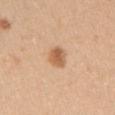<lesion>
<biopsy_status>not biopsied; imaged during a skin examination</biopsy_status>
<lesion_size>
  <long_diameter_mm_approx>3.0</long_diameter_mm_approx>
</lesion_size>
<site>left upper arm</site>
<lighting>white-light</lighting>
<image>
  <source>total-body photography crop</source>
  <field_of_view_mm>15</field_of_view_mm>
</image>
<patient>
  <sex>female</sex>
  <age_approx>35</age_approx>
</patient>
</lesion>